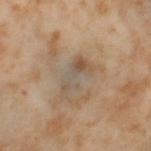Notes:
* biopsy status: catalogued during a skin exam; not biopsied
* location: the left thigh
* patient: female, aged around 55
* imaging modality: total-body-photography crop, ~15 mm field of view